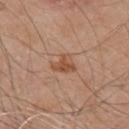Assessment:
This lesion was catalogued during total-body skin photography and was not selected for biopsy.
Background:
A roughly 15 mm field-of-view crop from a total-body skin photograph. The total-body-photography lesion software estimated an area of roughly 5 mm², an outline eccentricity of about 0.55 (0 = round, 1 = elongated), and a symmetry-axis asymmetry near 0.4. The analysis additionally found a mean CIELAB color near L≈51 a*≈22 b*≈33, about 9 CIELAB-L* units darker than the surrounding skin, and a normalized border contrast of about 7. It also reported border irregularity of about 4 on a 0–10 scale and a within-lesion color-variation index near 3.5/10. The analysis additionally found a nevus-likeness score of about 80/100. From the front of the torso. Captured under white-light illumination. A male subject, about 80 years old.